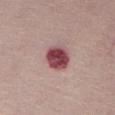| key | value |
|---|---|
| notes | imaged on a skin check; not biopsied |
| subject | female, roughly 50 years of age |
| location | the chest |
| imaging modality | ~15 mm tile from a whole-body skin photo |